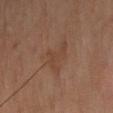  biopsy_status: not biopsied; imaged during a skin examination
  site: chest
  lighting: cross-polarized
  patient:
    sex: male
    age_approx: 60
  lesion_size:
    long_diameter_mm_approx: 4.0
  image:
    source: total-body photography crop
    field_of_view_mm: 15
  automated_metrics:
    eccentricity: 0.65
    border_irregularity_0_10: 6.5
    color_variation_0_10: 1.5
    peripheral_color_asymmetry: 0.5